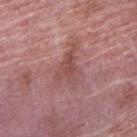workup = total-body-photography surveillance lesion; no biopsy.